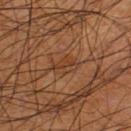biopsy status — total-body-photography surveillance lesion; no biopsy
subject — male, in their 60s
automated metrics — a lesion area of about 4 mm² and an eccentricity of roughly 0.8; a border-irregularity rating of about 4.5/10, a color-variation rating of about 3/10, and a peripheral color-asymmetry measure near 1; an automated nevus-likeness rating near 5 out of 100 and lesion-presence confidence of about 90/100
diameter — about 3 mm
image source — ~15 mm crop, total-body skin-cancer survey
site — the right lower leg